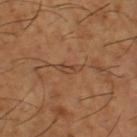follow-up = catalogued during a skin exam; not biopsied
acquisition = total-body-photography crop, ~15 mm field of view
site = the leg
patient = male, aged 63 to 67